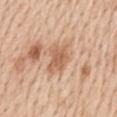Case summary:
* workup — catalogued during a skin exam; not biopsied
* location — the mid back
* diameter — about 4 mm
* automated metrics — a shape eccentricity near 0.8 and a symmetry-axis asymmetry near 0.25; a classifier nevus-likeness of about 0/100 and a detector confidence of about 100 out of 100 that the crop contains a lesion
* subject — male, roughly 60 years of age
* imaging modality — ~15 mm tile from a whole-body skin photo
* illumination — white-light illumination